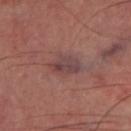- workup — total-body-photography surveillance lesion; no biopsy
- subject — male, about 65 years old
- imaging modality — ~15 mm crop, total-body skin-cancer survey
- location — the leg
- illumination — cross-polarized illumination